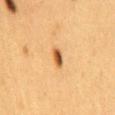Part of a total-body skin-imaging series; this lesion was reviewed on a skin check and was not flagged for biopsy.
A female subject, aged approximately 40.
Automated tile analysis of the lesion measured an average lesion color of about L≈47 a*≈21 b*≈37 (CIELAB).
From the mid back.
Imaged with cross-polarized lighting.
Longest diameter approximately 3 mm.
A close-up tile cropped from a whole-body skin photograph, about 15 mm across.